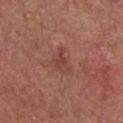No biopsy was performed on this lesion — it was imaged during a full skin examination and was not determined to be concerning.
A 15 mm close-up extracted from a 3D total-body photography capture.
This is a white-light tile.
About 3 mm across.
An algorithmic analysis of the crop reported a lesion area of about 3.5 mm² and an outline eccentricity of about 0.85 (0 = round, 1 = elongated). It also reported a color-variation rating of about 2/10 and radial color variation of about 0.5. It also reported a nevus-likeness score of about 0/100 and lesion-presence confidence of about 100/100.
The patient is a female aged approximately 65.
From the chest.